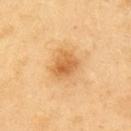| field | value |
|---|---|
| workup | no biopsy performed (imaged during a skin exam) |
| image source | ~15 mm crop, total-body skin-cancer survey |
| subject | male, approximately 60 years of age |
| body site | the arm |